The lesion-visualizer software estimated a footprint of about 15 mm², a shape eccentricity near 0.85, and a shape-asymmetry score of about 0.45 (0 = symmetric). The analysis additionally found a border-irregularity rating of about 6/10, a within-lesion color-variation index near 4.5/10, and radial color variation of about 1.5 · the tile uses white-light illumination · the patient is a male aged approximately 60 · from the chest · cropped from a total-body skin-imaging series; the visible field is about 15 mm · longest diameter approximately 6.5 mm:
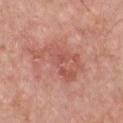Q: What did pathology find?
A: a seborrheic keratosis (benign)